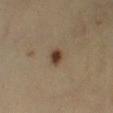Clinical impression: No biopsy was performed on this lesion — it was imaged during a full skin examination and was not determined to be concerning. Image and clinical context: Imaged with cross-polarized lighting. On the leg. A 15 mm crop from a total-body photograph taken for skin-cancer surveillance. Automated image analysis of the tile measured a footprint of about 3.5 mm² and a shape-asymmetry score of about 0.35 (0 = symmetric). The software also gave a lesion color around L≈35 a*≈13 b*≈24 in CIELAB, about 11 CIELAB-L* units darker than the surrounding skin, and a lesion-to-skin contrast of about 10.5 (normalized; higher = more distinct). And it measured a color-variation rating of about 4/10. It also reported a nevus-likeness score of about 100/100. Measured at roughly 2.5 mm in maximum diameter. A male subject, approximately 35 years of age.